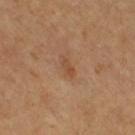* workup · total-body-photography surveillance lesion; no biopsy
* tile lighting · cross-polarized illumination
* acquisition · ~15 mm crop, total-body skin-cancer survey
* site · the right thigh
* patient · female, roughly 55 years of age
* lesion size · ~3 mm (longest diameter)